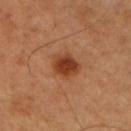Findings:
• notes — no biopsy performed (imaged during a skin exam)
• automated lesion analysis — a lesion area of about 7.5 mm²; a mean CIELAB color near L≈41 a*≈26 b*≈35, a lesion–skin lightness drop of about 12, and a normalized border contrast of about 9.5; internal color variation of about 4 on a 0–10 scale and a peripheral color-asymmetry measure near 1
• tile lighting — cross-polarized illumination
• acquisition — ~15 mm crop, total-body skin-cancer survey
• patient — male, in their 50s
• location — the right upper arm